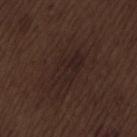{"lesion_size": {"long_diameter_mm_approx": 4.5}, "patient": {"sex": "male", "age_approx": 70}, "lighting": "white-light", "image": {"source": "total-body photography crop", "field_of_view_mm": 15}, "site": "back", "automated_metrics": {"area_mm2_approx": 9.5, "eccentricity": 0.8, "shape_asymmetry": 0.2, "border_irregularity_0_10": 3.0, "color_variation_0_10": 3.0, "peripheral_color_asymmetry": 1.0}}